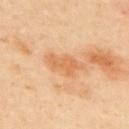Image and clinical context: The lesion is located on the upper back. The subject is a male aged 48–52. Captured under cross-polarized illumination. A 15 mm crop from a total-body photograph taken for skin-cancer surveillance.